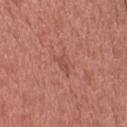No biopsy was performed on this lesion — it was imaged during a full skin examination and was not determined to be concerning.
The lesion is on the head or neck.
A 15 mm crop from a total-body photograph taken for skin-cancer surveillance.
The tile uses white-light illumination.
An algorithmic analysis of the crop reported a classifier nevus-likeness of about 0/100.
A male subject, aged 48 to 52.